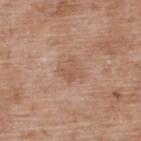notes=imaged on a skin check; not biopsied
acquisition=total-body-photography crop, ~15 mm field of view
site=the upper back
patient=male, aged approximately 50
lesion size=about 3 mm
automated metrics=a border-irregularity index near 2.5/10, internal color variation of about 2 on a 0–10 scale, and a peripheral color-asymmetry measure near 1